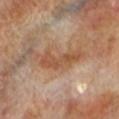Impression: Recorded during total-body skin imaging; not selected for excision or biopsy. Acquisition and patient details: The subject is a male aged 68–72. This is a cross-polarized tile. The lesion is on the left lower leg. A region of skin cropped from a whole-body photographic capture, roughly 15 mm wide.